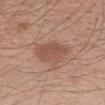Impression: Part of a total-body skin-imaging series; this lesion was reviewed on a skin check and was not flagged for biopsy. Clinical summary: The lesion is on the left forearm. A male patient, approximately 60 years of age. Cropped from a total-body skin-imaging series; the visible field is about 15 mm.